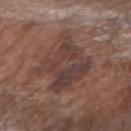notes: total-body-photography surveillance lesion; no biopsy
patient: male, aged approximately 70
site: the right forearm
image source: ~15 mm tile from a whole-body skin photo